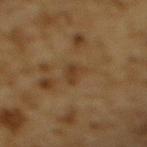Part of a total-body skin-imaging series; this lesion was reviewed on a skin check and was not flagged for biopsy. This is a cross-polarized tile. Measured at roughly 3 mm in maximum diameter. A male subject approximately 85 years of age or older. Located on the upper back. A close-up tile cropped from a whole-body skin photograph, about 15 mm across.